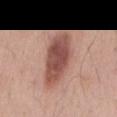{"biopsy_status": "not biopsied; imaged during a skin examination", "lighting": "white-light", "lesion_size": {"long_diameter_mm_approx": 8.0}, "site": "back", "patient": {"sex": "male", "age_approx": 55}, "image": {"source": "total-body photography crop", "field_of_view_mm": 15}}